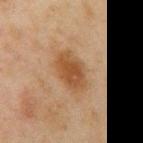Acquisition and patient details:
The lesion-visualizer software estimated an average lesion color of about L≈40 a*≈17 b*≈31 (CIELAB) and a normalized lesion–skin contrast near 8.5. The software also gave a color-variation rating of about 4/10. The tile uses cross-polarized illumination. The lesion is on the right upper arm. The recorded lesion diameter is about 4.5 mm. Cropped from a total-body skin-imaging series; the visible field is about 15 mm. The patient is a male in their mid-40s.